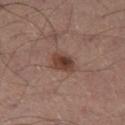| feature | finding |
|---|---|
| biopsy status | total-body-photography surveillance lesion; no biopsy |